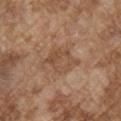Q: Is there a histopathology result?
A: no biopsy performed (imaged during a skin exam)
Q: How was the tile lit?
A: white-light
Q: What are the patient's age and sex?
A: male, roughly 75 years of age
Q: What is the imaging modality?
A: ~15 mm tile from a whole-body skin photo
Q: What is the lesion's diameter?
A: ≈5 mm
Q: What is the anatomic site?
A: the right upper arm